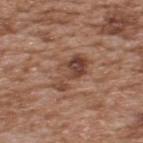The lesion was photographed on a routine skin check and not biopsied; there is no pathology result. A male subject aged 63–67. A roughly 15 mm field-of-view crop from a total-body skin photograph. From the upper back.